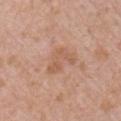Clinical impression: Part of a total-body skin-imaging series; this lesion was reviewed on a skin check and was not flagged for biopsy. Acquisition and patient details: On the right upper arm. A 15 mm crop from a total-body photograph taken for skin-cancer surveillance. A male patient, in their 50s. The tile uses white-light illumination. Measured at roughly 4 mm in maximum diameter.